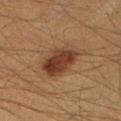<tbp_lesion>
<image>
  <source>total-body photography crop</source>
  <field_of_view_mm>15</field_of_view_mm>
</image>
<automated_metrics>
  <area_mm2_approx>15.0</area_mm2_approx>
  <shape_asymmetry>0.2</shape_asymmetry>
  <cielab_L>31</cielab_L>
  <cielab_a>17</cielab_a>
  <cielab_b>25</cielab_b>
</automated_metrics>
<patient>
  <sex>male</sex>
  <age_approx>50</age_approx>
</patient>
<site>left lower leg</site>
<lighting>cross-polarized</lighting>
</tbp_lesion>